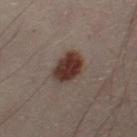Automated image analysis of the tile measured an average lesion color of about L≈29 a*≈16 b*≈19 (CIELAB) and about 13 CIELAB-L* units darker than the surrounding skin. And it measured border irregularity of about 1.5 on a 0–10 scale and peripheral color asymmetry of about 1.5. It also reported a nevus-likeness score of about 100/100 and lesion-presence confidence of about 100/100. The patient is a male aged approximately 50. A 15 mm close-up tile from a total-body photography series done for melanoma screening. On the leg. Approximately 3.5 mm at its widest.